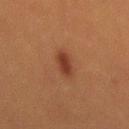Notes:
– notes — catalogued during a skin exam; not biopsied
– subject — male, in their 40s
– body site — the mid back
– lesion size — ≈3.5 mm
– image source — total-body-photography crop, ~15 mm field of view